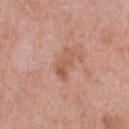Q: Was this lesion biopsied?
A: no biopsy performed (imaged during a skin exam)
Q: What are the patient's age and sex?
A: male, about 50 years old
Q: What is the lesion's diameter?
A: ≈3.5 mm
Q: What lighting was used for the tile?
A: white-light illumination
Q: How was this image acquired?
A: total-body-photography crop, ~15 mm field of view
Q: What is the anatomic site?
A: the chest